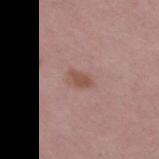workup: no biopsy performed (imaged during a skin exam)
anatomic site: the right thigh
automated metrics: a lesion color around L≈51 a*≈21 b*≈25 in CIELAB and a normalized lesion–skin contrast near 7; a classifier nevus-likeness of about 45/100 and a lesion-detection confidence of about 100/100
subject: female, about 40 years old
acquisition: total-body-photography crop, ~15 mm field of view